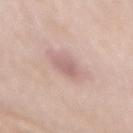lighting: white-light
site: back
lesion_size:
  long_diameter_mm_approx: 2.5
image:
  source: total-body photography crop
  field_of_view_mm: 15
patient:
  sex: female
  age_approx: 60